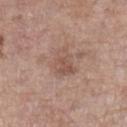Q: Was a biopsy performed?
A: total-body-photography surveillance lesion; no biopsy
Q: Lesion size?
A: ~3.5 mm (longest diameter)
Q: Illumination type?
A: white-light
Q: What is the imaging modality?
A: ~15 mm tile from a whole-body skin photo
Q: Automated lesion metrics?
A: a mean CIELAB color near L≈53 a*≈18 b*≈26, about 8 CIELAB-L* units darker than the surrounding skin, and a normalized border contrast of about 5.5; a classifier nevus-likeness of about 0/100
Q: Patient demographics?
A: female, aged approximately 85 or older
Q: Lesion location?
A: the left lower leg